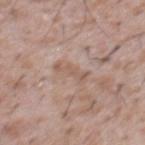Case summary:
– workup — imaged on a skin check; not biopsied
– diameter — about 3.5 mm
– lighting — white-light illumination
– subject — male, in their mid- to late 40s
– image — 15 mm crop, total-body photography
– anatomic site — the chest